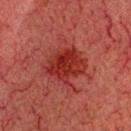subject = male, roughly 60 years of age | location = the head or neck | TBP lesion metrics = an area of roughly 15 mm²; roughly 8 lightness units darker than nearby skin; internal color variation of about 4 on a 0–10 scale and peripheral color asymmetry of about 1; an automated nevus-likeness rating near 30 out of 100 | lesion diameter = ≈4.5 mm | tile lighting = cross-polarized | acquisition = ~15 mm crop, total-body skin-cancer survey.